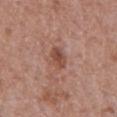Q: Is there a histopathology result?
A: total-body-photography surveillance lesion; no biopsy
Q: Where on the body is the lesion?
A: the front of the torso
Q: How was the tile lit?
A: white-light
Q: Lesion size?
A: ≈3 mm
Q: What kind of image is this?
A: 15 mm crop, total-body photography
Q: What are the patient's age and sex?
A: male, aged 63 to 67